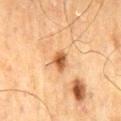Recorded during total-body skin imaging; not selected for excision or biopsy. From the mid back. A male subject aged approximately 70. About 2.5 mm across. A close-up tile cropped from a whole-body skin photograph, about 15 mm across.